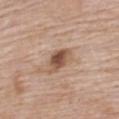Q: Was this lesion biopsied?
A: total-body-photography surveillance lesion; no biopsy
Q: What are the patient's age and sex?
A: female, aged 68 to 72
Q: What is the imaging modality?
A: ~15 mm crop, total-body skin-cancer survey
Q: Lesion location?
A: the upper back